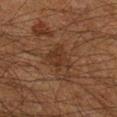| key | value |
|---|---|
| follow-up | total-body-photography surveillance lesion; no biopsy |
| automated lesion analysis | a mean CIELAB color near L≈25 a*≈15 b*≈23, roughly 5 lightness units darker than nearby skin, and a normalized border contrast of about 6 |
| image | ~15 mm tile from a whole-body skin photo |
| lesion size | ~3.5 mm (longest diameter) |
| patient | male, aged 58–62 |
| tile lighting | cross-polarized |
| location | the right lower leg |